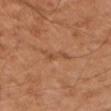Captured during whole-body skin photography for melanoma surveillance; the lesion was not biopsied.
A 15 mm close-up extracted from a 3D total-body photography capture.
About 3 mm across.
The tile uses cross-polarized illumination.
Located on the left upper arm.
A male patient aged around 55.